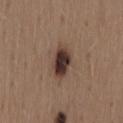Part of a total-body skin-imaging series; this lesion was reviewed on a skin check and was not flagged for biopsy. Approximately 4 mm at its widest. Automated tile analysis of the lesion measured an area of roughly 7.5 mm², an outline eccentricity of about 0.8 (0 = round, 1 = elongated), and two-axis asymmetry of about 0.2. The analysis additionally found a border-irregularity index near 2/10, a within-lesion color-variation index near 5/10, and a peripheral color-asymmetry measure near 1.5. And it measured a detector confidence of about 100 out of 100 that the crop contains a lesion. From the mid back. This image is a 15 mm lesion crop taken from a total-body photograph. A female patient aged approximately 40.